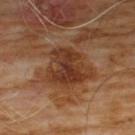The lesion was tiled from a total-body skin photograph and was not biopsied. This is a cross-polarized tile. A male patient, approximately 60 years of age. Measured at roughly 5.5 mm in maximum diameter. The lesion is located on the front of the torso. A region of skin cropped from a whole-body photographic capture, roughly 15 mm wide.